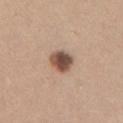| key | value |
|---|---|
| follow-up | imaged on a skin check; not biopsied |
| size | ≈3 mm |
| TBP lesion metrics | an area of roughly 6.5 mm², an eccentricity of roughly 0.5, and two-axis asymmetry of about 0.2; about 17 CIELAB-L* units darker than the surrounding skin and a lesion-to-skin contrast of about 11.5 (normalized; higher = more distinct); a border-irregularity index near 1.5/10, a within-lesion color-variation index near 6/10, and peripheral color asymmetry of about 1.5; an automated nevus-likeness rating near 95 out of 100 and a detector confidence of about 100 out of 100 that the crop contains a lesion |
| patient | female, aged 23–27 |
| anatomic site | the left upper arm |
| image | ~15 mm crop, total-body skin-cancer survey |